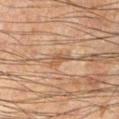| field | value |
|---|---|
| workup | total-body-photography surveillance lesion; no biopsy |
| imaging modality | ~15 mm tile from a whole-body skin photo |
| subject | male, approximately 60 years of age |
| location | the left leg |
| size | about 3 mm |